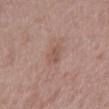<record>
  <image>
    <source>total-body photography crop</source>
    <field_of_view_mm>15</field_of_view_mm>
  </image>
  <lighting>white-light</lighting>
  <patient>
    <sex>female</sex>
    <age_approx>45</age_approx>
  </patient>
  <lesion_size>
    <long_diameter_mm_approx>3.0</long_diameter_mm_approx>
  </lesion_size>
  <site>right upper arm</site>
</record>